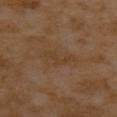notes = total-body-photography surveillance lesion; no biopsy | body site = the upper back | patient = female, approximately 55 years of age | acquisition = ~15 mm crop, total-body skin-cancer survey.